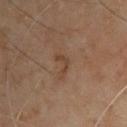No biopsy was performed on this lesion — it was imaged during a full skin examination and was not determined to be concerning. The lesion is on the chest. The total-body-photography lesion software estimated an average lesion color of about L≈42 a*≈18 b*≈30 (CIELAB), a lesion–skin lightness drop of about 7, and a normalized lesion–skin contrast near 6.5. And it measured a classifier nevus-likeness of about 0/100. A male subject, aged approximately 65. Approximately 2.5 mm at its widest. Captured under cross-polarized illumination. A 15 mm crop from a total-body photograph taken for skin-cancer surveillance.